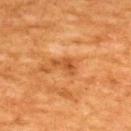Recorded during total-body skin imaging; not selected for excision or biopsy. Located on the upper back. A close-up tile cropped from a whole-body skin photograph, about 15 mm across. A male subject approximately 60 years of age.